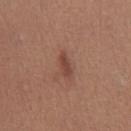<record>
<biopsy_status>not biopsied; imaged during a skin examination</biopsy_status>
</record>